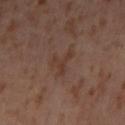Clinical impression:
No biopsy was performed on this lesion — it was imaged during a full skin examination and was not determined to be concerning.
Acquisition and patient details:
The lesion's longest dimension is about 4 mm. The lesion is on the left thigh. This is a cross-polarized tile. A roughly 15 mm field-of-view crop from a total-body skin photograph. The patient is a female aged approximately 55.